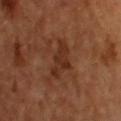  biopsy_status: not biopsied; imaged during a skin examination
  site: upper back
  lesion_size:
    long_diameter_mm_approx: 4.5
  image:
    source: total-body photography crop
    field_of_view_mm: 15